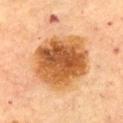  image:
    source: total-body photography crop
    field_of_view_mm: 15
  lighting: cross-polarized
  patient:
    sex: female
    age_approx: 60
  site: chest
  automated_metrics:
    cielab_L: 52
    cielab_a: 23
    cielab_b: 40
    vs_skin_contrast_norm: 11.0
  lesion_size:
    long_diameter_mm_approx: 7.5